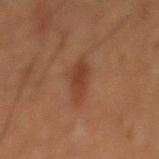Q: Is there a histopathology result?
A: imaged on a skin check; not biopsied
Q: What kind of image is this?
A: ~15 mm tile from a whole-body skin photo
Q: How large is the lesion?
A: about 5 mm
Q: What are the patient's age and sex?
A: male, in their 60s
Q: Where on the body is the lesion?
A: the abdomen
Q: How was the tile lit?
A: cross-polarized illumination